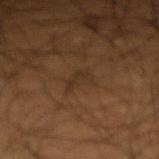The lesion was photographed on a routine skin check and not biopsied; there is no pathology result. A male patient aged around 50. This image is a 15 mm lesion crop taken from a total-body photograph. Located on the right forearm.